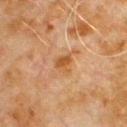{
  "patient": {
    "sex": "male",
    "age_approx": 80
  },
  "lighting": "cross-polarized",
  "lesion_size": {
    "long_diameter_mm_approx": 2.5
  },
  "image": {
    "source": "total-body photography crop",
    "field_of_view_mm": 15
  },
  "automated_metrics": {
    "border_irregularity_0_10": 2.5,
    "color_variation_0_10": 3.5,
    "peripheral_color_asymmetry": 1.0,
    "nevus_likeness_0_100": 10,
    "lesion_detection_confidence_0_100": 100
  },
  "site": "chest"
}